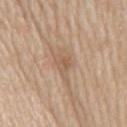Clinical impression:
Imaged during a routine full-body skin examination; the lesion was not biopsied and no histopathology is available.
Clinical summary:
A region of skin cropped from a whole-body photographic capture, roughly 15 mm wide. The total-body-photography lesion software estimated a shape eccentricity near 0.9 and a symmetry-axis asymmetry near 0.4. The analysis additionally found a color-variation rating of about 2.5/10 and peripheral color asymmetry of about 0.5. On the mid back. Imaged with white-light lighting. The subject is a male aged approximately 60. The recorded lesion diameter is about 4.5 mm.